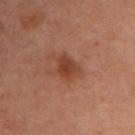{"biopsy_status": "not biopsied; imaged during a skin examination", "patient": {"sex": "female", "age_approx": 55}, "automated_metrics": {"area_mm2_approx": 5.0, "eccentricity": 0.6, "shape_asymmetry": 0.25, "cielab_L": 34, "cielab_a": 21, "cielab_b": 27, "vs_skin_darker_L": 8.0, "vs_skin_contrast_norm": 7.5, "nevus_likeness_0_100": 65, "lesion_detection_confidence_0_100": 100}, "site": "chest", "image": {"source": "total-body photography crop", "field_of_view_mm": 15}, "lesion_size": {"long_diameter_mm_approx": 3.0}, "lighting": "cross-polarized"}